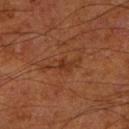follow-up: total-body-photography surveillance lesion; no biopsy
size: ~4 mm (longest diameter)
imaging modality: ~15 mm crop, total-body skin-cancer survey
patient: male, roughly 65 years of age
site: the leg
lighting: cross-polarized